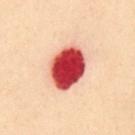Impression: The lesion was photographed on a routine skin check and not biopsied; there is no pathology result. Background: On the abdomen. A close-up tile cropped from a whole-body skin photograph, about 15 mm across. Automated tile analysis of the lesion measured a mean CIELAB color near L≈44 a*≈40 b*≈29, about 27 CIELAB-L* units darker than the surrounding skin, and a normalized border contrast of about 17. The software also gave a border-irregularity index near 1/10 and peripheral color asymmetry of about 1. It also reported a nevus-likeness score of about 0/100 and a lesion-detection confidence of about 100/100. The patient is a female approximately 60 years of age. Captured under cross-polarized illumination.